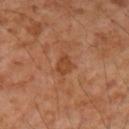Impression: The lesion was tiled from a total-body skin photograph and was not biopsied. Image and clinical context: A male patient aged 53 to 57. On the right forearm. This image is a 15 mm lesion crop taken from a total-body photograph.